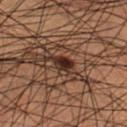Imaged during a routine full-body skin examination; the lesion was not biopsied and no histopathology is available.
Measured at roughly 2.5 mm in maximum diameter.
From the right lower leg.
Imaged with cross-polarized lighting.
A male patient approximately 50 years of age.
Automated image analysis of the tile measured a lesion area of about 4 mm², an outline eccentricity of about 0.55 (0 = round, 1 = elongated), and two-axis asymmetry of about 0.25. The analysis additionally found an automated nevus-likeness rating near 95 out of 100 and a lesion-detection confidence of about 95/100.
A lesion tile, about 15 mm wide, cut from a 3D total-body photograph.